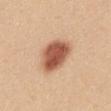biopsy status=total-body-photography surveillance lesion; no biopsy | imaging modality=~15 mm tile from a whole-body skin photo | tile lighting=white-light illumination | size=~5 mm (longest diameter) | body site=the back | patient=female, aged approximately 25.